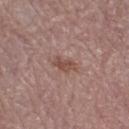<lesion>
<automated_metrics>
  <area_mm2_approx>4.0</area_mm2_approx>
  <shape_asymmetry>0.35</shape_asymmetry>
  <border_irregularity_0_10>3.5</border_irregularity_0_10>
</automated_metrics>
<image>
  <source>total-body photography crop</source>
  <field_of_view_mm>15</field_of_view_mm>
</image>
<patient>
  <sex>female</sex>
  <age_approx>65</age_approx>
</patient>
<lighting>white-light</lighting>
<site>leg</site>
</lesion>